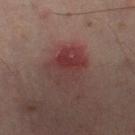Imaged during a routine full-body skin examination; the lesion was not biopsied and no histopathology is available.